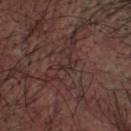{
  "biopsy_status": "not biopsied; imaged during a skin examination",
  "site": "head or neck",
  "image": {
    "source": "total-body photography crop",
    "field_of_view_mm": 15
  },
  "patient": {
    "sex": "male",
    "age_approx": 70
  }
}